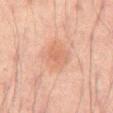Captured during whole-body skin photography for melanoma surveillance; the lesion was not biopsied. The lesion is on the mid back. A male subject aged 63 to 67. A region of skin cropped from a whole-body photographic capture, roughly 15 mm wide. Imaged with cross-polarized lighting. An algorithmic analysis of the crop reported a border-irregularity rating of about 2/10, internal color variation of about 2.5 on a 0–10 scale, and a peripheral color-asymmetry measure near 1. The software also gave a classifier nevus-likeness of about 10/100 and a lesion-detection confidence of about 100/100. The lesion's longest dimension is about 4 mm.